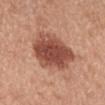  patient:
    sex: female
    age_approx: 40
  image:
    source: total-body photography crop
    field_of_view_mm: 15
  automated_metrics:
    area_mm2_approx: 22.0
    eccentricity: 0.6
  site: back
  lesion_size:
    long_diameter_mm_approx: 6.0
  lighting: white-light